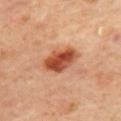Captured under cross-polarized illumination. A female patient, roughly 45 years of age. An algorithmic analysis of the crop reported a border-irregularity index near 1.5/10 and peripheral color asymmetry of about 2.5. And it measured a classifier nevus-likeness of about 100/100 and a lesion-detection confidence of about 100/100. Located on the mid back. Longest diameter approximately 4 mm. A region of skin cropped from a whole-body photographic capture, roughly 15 mm wide.